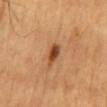Recorded during total-body skin imaging; not selected for excision or biopsy. Measured at roughly 3 mm in maximum diameter. Captured under cross-polarized illumination. A male patient, aged approximately 65. The lesion is located on the mid back. A close-up tile cropped from a whole-body skin photograph, about 15 mm across.